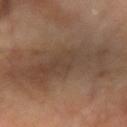Captured during whole-body skin photography for melanoma surveillance; the lesion was not biopsied. Captured under cross-polarized illumination. A male patient, aged approximately 70. A 15 mm close-up extracted from a 3D total-body photography capture. The lesion is located on the left forearm. About 12 mm across.